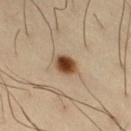The total-body-photography lesion software estimated a classifier nevus-likeness of about 100/100 and a lesion-detection confidence of about 100/100. The tile uses cross-polarized illumination. Longest diameter approximately 2.5 mm. A 15 mm crop from a total-body photograph taken for skin-cancer surveillance. The lesion is on the right thigh. The subject is a male roughly 35 years of age.